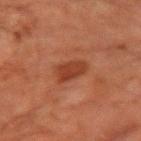Clinical impression: The lesion was tiled from a total-body skin photograph and was not biopsied. Background: The lesion is on the arm. A male patient in their 70s. The recorded lesion diameter is about 4 mm. Cropped from a whole-body photographic skin survey; the tile spans about 15 mm.